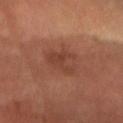Findings:
- biopsy status: total-body-photography surveillance lesion; no biopsy
- lesion size: ~4 mm (longest diameter)
- image source: ~15 mm tile from a whole-body skin photo
- lighting: cross-polarized illumination
- patient: male, in their mid-50s
- anatomic site: the head or neck
- automated lesion analysis: a mean CIELAB color near L≈37 a*≈22 b*≈27 and a normalized lesion–skin contrast near 5.5; a border-irregularity rating of about 5.5/10 and radial color variation of about 0.5; an automated nevus-likeness rating near 0 out of 100 and a lesion-detection confidence of about 100/100